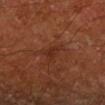notes: catalogued during a skin exam; not biopsied | diameter: about 3 mm | image-analysis metrics: a mean CIELAB color near L≈28 a*≈23 b*≈28 and about 6 CIELAB-L* units darker than the surrounding skin; a classifier nevus-likeness of about 0/100 and a detector confidence of about 90 out of 100 that the crop contains a lesion | patient: male, aged approximately 65 | tile lighting: cross-polarized illumination | location: the left lower leg | image: total-body-photography crop, ~15 mm field of view.